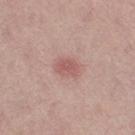Assessment:
The lesion was photographed on a routine skin check and not biopsied; there is no pathology result.
Acquisition and patient details:
The patient is a female roughly 65 years of age. The recorded lesion diameter is about 3 mm. A close-up tile cropped from a whole-body skin photograph, about 15 mm across. Located on the left thigh. The tile uses white-light illumination.